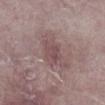Recorded during total-body skin imaging; not selected for excision or biopsy.
From the right lower leg.
Captured under white-light illumination.
The subject is a female aged approximately 60.
Measured at roughly 2.5 mm in maximum diameter.
A lesion tile, about 15 mm wide, cut from a 3D total-body photograph.
Automated image analysis of the tile measured an average lesion color of about L≈48 a*≈19 b*≈16 (CIELAB), roughly 7 lightness units darker than nearby skin, and a normalized border contrast of about 5.5. The analysis additionally found a classifier nevus-likeness of about 0/100 and a detector confidence of about 95 out of 100 that the crop contains a lesion.